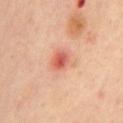location — the chest
patient — female, in their mid- to late 40s
imaging modality — total-body-photography crop, ~15 mm field of view